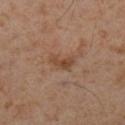Impression:
Part of a total-body skin-imaging series; this lesion was reviewed on a skin check and was not flagged for biopsy.